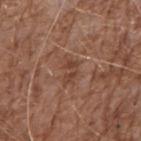notes = total-body-photography surveillance lesion; no biopsy | subject = male, approximately 70 years of age | illumination = white-light illumination | automated metrics = an area of roughly 3.5 mm², a shape eccentricity near 0.85, and a symmetry-axis asymmetry near 0.5; a mean CIELAB color near L≈42 a*≈21 b*≈28, a lesion–skin lightness drop of about 7, and a normalized border contrast of about 6; a border-irregularity index near 6/10, a color-variation rating of about 0/10, and a peripheral color-asymmetry measure near 0; a classifier nevus-likeness of about 0/100 | body site = the right upper arm | imaging modality = total-body-photography crop, ~15 mm field of view | size = ≈3 mm.